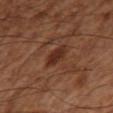<tbp_lesion>
<biopsy_status>not biopsied; imaged during a skin examination</biopsy_status>
<lesion_size>
  <long_diameter_mm_approx>3.5</long_diameter_mm_approx>
</lesion_size>
<automated_metrics>
  <eccentricity>0.8</eccentricity>
  <cielab_L>30</cielab_L>
  <cielab_a>23</cielab_a>
  <cielab_b>28</cielab_b>
  <vs_skin_darker_L>9.0</vs_skin_darker_L>
  <vs_skin_contrast_norm>8.5</vs_skin_contrast_norm>
  <border_irregularity_0_10>3.0</border_irregularity_0_10>
  <color_variation_0_10>2.0</color_variation_0_10>
  <peripheral_color_asymmetry>0.5</peripheral_color_asymmetry>
  <nevus_likeness_0_100>40</nevus_likeness_0_100>
  <lesion_detection_confidence_0_100>100</lesion_detection_confidence_0_100>
</automated_metrics>
<image>
  <source>total-body photography crop</source>
  <field_of_view_mm>15</field_of_view_mm>
</image>
<lighting>cross-polarized</lighting>
<patient>
  <sex>male</sex>
  <age_approx>55</age_approx>
</patient>
<site>right thigh</site>
</tbp_lesion>